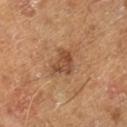* follow-up: imaged on a skin check; not biopsied
* patient: male, in their mid-60s
* size: ≈3.5 mm
* imaging modality: ~15 mm tile from a whole-body skin photo
* body site: the right lower leg
* lighting: cross-polarized illumination